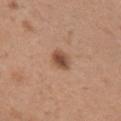Assessment:
Part of a total-body skin-imaging series; this lesion was reviewed on a skin check and was not flagged for biopsy.
Clinical summary:
The lesion is located on the arm. Cropped from a total-body skin-imaging series; the visible field is about 15 mm. Approximately 2.5 mm at its widest. An algorithmic analysis of the crop reported a border-irregularity rating of about 1/10, internal color variation of about 4.5 on a 0–10 scale, and peripheral color asymmetry of about 1.5. The analysis additionally found a classifier nevus-likeness of about 95/100. This is a white-light tile. A female patient aged approximately 30.